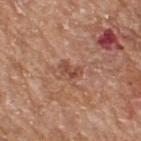  biopsy_status: not biopsied; imaged during a skin examination
  lighting: white-light
  image:
    source: total-body photography crop
    field_of_view_mm: 15
  lesion_size:
    long_diameter_mm_approx: 2.5
  site: upper back
  patient:
    sex: male
    age_approx: 65